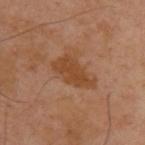Assessment: No biopsy was performed on this lesion — it was imaged during a full skin examination and was not determined to be concerning. Acquisition and patient details: Cropped from a whole-body photographic skin survey; the tile spans about 15 mm. Longest diameter approximately 5 mm. The tile uses cross-polarized illumination. A male patient, aged around 40. The lesion is located on the upper back.